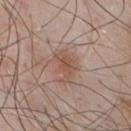Assessment:
This lesion was catalogued during total-body skin photography and was not selected for biopsy.
Image and clinical context:
Located on the chest. A close-up tile cropped from a whole-body skin photograph, about 15 mm across. A male subject in their mid- to late 50s.